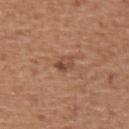Clinical impression: This lesion was catalogued during total-body skin photography and was not selected for biopsy. Context: This is a white-light tile. A roughly 15 mm field-of-view crop from a total-body skin photograph. The lesion's longest dimension is about 2.5 mm. From the arm. A male patient roughly 60 years of age.